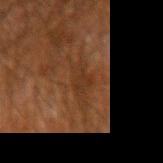| feature | finding |
|---|---|
| workup | total-body-photography surveillance lesion; no biopsy |
| anatomic site | the right forearm |
| acquisition | total-body-photography crop, ~15 mm field of view |
| lighting | cross-polarized |
| lesion size | ≈4.5 mm |
| subject | male, aged approximately 60 |